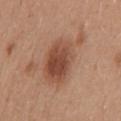Captured during whole-body skin photography for melanoma surveillance; the lesion was not biopsied.